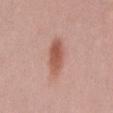Background: The tile uses white-light illumination. Longest diameter approximately 5 mm. A female subject aged approximately 25. On the mid back. A 15 mm close-up tile from a total-body photography series done for melanoma screening.